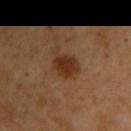| feature | finding |
|---|---|
| workup | catalogued during a skin exam; not biopsied |
| lighting | cross-polarized illumination |
| site | the right upper arm |
| diameter | ~4 mm (longest diameter) |
| image | ~15 mm tile from a whole-body skin photo |
| patient | male, aged 48–52 |